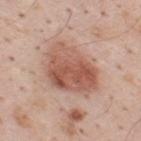No biopsy was performed on this lesion — it was imaged during a full skin examination and was not determined to be concerning.
A 15 mm close-up tile from a total-body photography series done for melanoma screening.
The lesion is on the upper back.
The patient is a male about 35 years old.